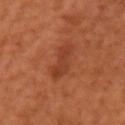Findings:
– workup: imaged on a skin check; not biopsied
– imaging modality: ~15 mm tile from a whole-body skin photo
– lighting: cross-polarized
– subject: female, aged approximately 65
– lesion size: ≈4 mm
– location: the right upper arm